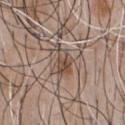The lesion was tiled from a total-body skin photograph and was not biopsied. The lesion-visualizer software estimated border irregularity of about 5.5 on a 0–10 scale, a color-variation rating of about 5.5/10, and a peripheral color-asymmetry measure near 2. A male subject, in their 50s. Approximately 4 mm at its widest. This image is a 15 mm lesion crop taken from a total-body photograph. The lesion is on the chest. The tile uses white-light illumination.